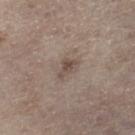Clinical impression:
Recorded during total-body skin imaging; not selected for excision or biopsy.
Clinical summary:
Cropped from a whole-body photographic skin survey; the tile spans about 15 mm. Located on the right lower leg. A male patient, in their 70s. The total-body-photography lesion software estimated an area of roughly 4 mm², a shape eccentricity near 0.8, and a symmetry-axis asymmetry near 0.5.